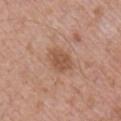| key | value |
|---|---|
| notes | imaged on a skin check; not biopsied |
| illumination | white-light |
| image | total-body-photography crop, ~15 mm field of view |
| size | about 3.5 mm |
| automated metrics | border irregularity of about 1.5 on a 0–10 scale, a within-lesion color-variation index near 2/10, and a peripheral color-asymmetry measure near 1 |
| patient | male, roughly 50 years of age |
| body site | the right upper arm |